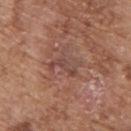workup: imaged on a skin check; not biopsied
location: the upper back
patient: male, aged 73 to 77
automated lesion analysis: a lesion color around L≈46 a*≈21 b*≈24 in CIELAB, roughly 7 lightness units darker than nearby skin, and a normalized border contrast of about 6
acquisition: ~15 mm crop, total-body skin-cancer survey
tile lighting: white-light illumination